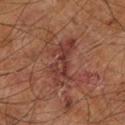Clinical impression: Part of a total-body skin-imaging series; this lesion was reviewed on a skin check and was not flagged for biopsy.